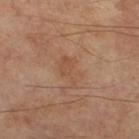Captured during whole-body skin photography for melanoma surveillance; the lesion was not biopsied.
An algorithmic analysis of the crop reported a mean CIELAB color near L≈48 a*≈20 b*≈31, a lesion–skin lightness drop of about 5, and a normalized lesion–skin contrast near 4.5. The analysis additionally found a classifier nevus-likeness of about 0/100 and a lesion-detection confidence of about 100/100.
The tile uses cross-polarized illumination.
About 4 mm across.
Located on the right thigh.
The patient is a male roughly 70 years of age.
A roughly 15 mm field-of-view crop from a total-body skin photograph.